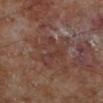<record>
  <biopsy_status>not biopsied; imaged during a skin examination</biopsy_status>
  <patient>
    <sex>male</sex>
    <age_approx>70</age_approx>
  </patient>
  <lighting>cross-polarized</lighting>
  <image>
    <source>total-body photography crop</source>
    <field_of_view_mm>15</field_of_view_mm>
  </image>
  <site>left lower leg</site>
  <lesion_size>
    <long_diameter_mm_approx>4.5</long_diameter_mm_approx>
  </lesion_size>
</record>